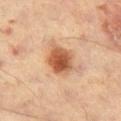Context: Cropped from a whole-body photographic skin survey; the tile spans about 15 mm. Captured under cross-polarized illumination. Approximately 4 mm at its widest. A male patient aged 58–62. The lesion-visualizer software estimated a footprint of about 9.5 mm², a shape eccentricity near 0.6, and two-axis asymmetry of about 0.15. The software also gave border irregularity of about 1.5 on a 0–10 scale, internal color variation of about 5.5 on a 0–10 scale, and radial color variation of about 1.5. The lesion is located on the leg.